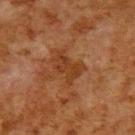Assessment:
Part of a total-body skin-imaging series; this lesion was reviewed on a skin check and was not flagged for biopsy.
Acquisition and patient details:
Captured under cross-polarized illumination. Approximately 4 mm at its widest. Cropped from a total-body skin-imaging series; the visible field is about 15 mm. The lesion is on the upper back. A male patient in their 80s.